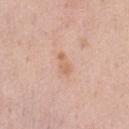<lesion>
  <biopsy_status>not biopsied; imaged during a skin examination</biopsy_status>
  <patient>
    <sex>female</sex>
    <age_approx>50</age_approx>
  </patient>
  <lesion_size>
    <long_diameter_mm_approx>2.5</long_diameter_mm_approx>
  </lesion_size>
  <site>left thigh</site>
  <lighting>white-light</lighting>
  <image>
    <source>total-body photography crop</source>
    <field_of_view_mm>15</field_of_view_mm>
  </image>
</lesion>